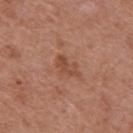Notes:
– follow-up — imaged on a skin check; not biopsied
– patient — male, approximately 70 years of age
– site — the mid back
– image — 15 mm crop, total-body photography
– TBP lesion metrics — a lesion color around L≈47 a*≈24 b*≈31 in CIELAB, about 8 CIELAB-L* units darker than the surrounding skin, and a normalized border contrast of about 6; a border-irregularity index near 4.5/10, internal color variation of about 1 on a 0–10 scale, and peripheral color asymmetry of about 0.5